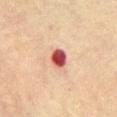Imaged during a routine full-body skin examination; the lesion was not biopsied and no histopathology is available.
This image is a 15 mm lesion crop taken from a total-body photograph.
The lesion is located on the abdomen.
An algorithmic analysis of the crop reported a lesion area of about 5 mm², a shape eccentricity near 0.6, and a shape-asymmetry score of about 0.35 (0 = symmetric). And it measured border irregularity of about 2.5 on a 0–10 scale, a color-variation rating of about 5.5/10, and peripheral color asymmetry of about 1.5.
A male patient, aged approximately 70.